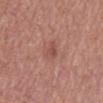Notes:
• notes · catalogued during a skin exam; not biopsied
• imaging modality · 15 mm crop, total-body photography
• anatomic site · the mid back
• patient · male, roughly 65 years of age